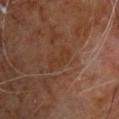Impression:
Captured during whole-body skin photography for melanoma surveillance; the lesion was not biopsied.
Acquisition and patient details:
An algorithmic analysis of the crop reported a lesion area of about 4.5 mm². This image is a 15 mm lesion crop taken from a total-body photograph. A male subject about 60 years old. On the chest. The lesion's longest dimension is about 4.5 mm.